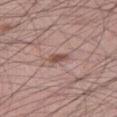<lesion>
<biopsy_status>not biopsied; imaged during a skin examination</biopsy_status>
<patient>
  <sex>male</sex>
  <age_approx>45</age_approx>
</patient>
<lighting>white-light</lighting>
<automated_metrics>
  <cielab_L>51</cielab_L>
  <cielab_a>20</cielab_a>
  <cielab_b>23</cielab_b>
  <vs_skin_darker_L>11.0</vs_skin_darker_L>
  <vs_skin_contrast_norm>7.5</vs_skin_contrast_norm>
  <border_irregularity_0_10>3.0</border_irregularity_0_10>
  <color_variation_0_10>1.5</color_variation_0_10>
</automated_metrics>
<lesion_size>
  <long_diameter_mm_approx>2.5</long_diameter_mm_approx>
</lesion_size>
<site>right thigh</site>
<image>
  <source>total-body photography crop</source>
  <field_of_view_mm>15</field_of_view_mm>
</image>
</lesion>